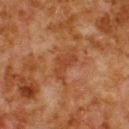Captured during whole-body skin photography for melanoma surveillance; the lesion was not biopsied.
A male patient roughly 80 years of age.
The tile uses cross-polarized illumination.
Approximately 4.5 mm at its widest.
The total-body-photography lesion software estimated internal color variation of about 2.5 on a 0–10 scale and radial color variation of about 1.
The lesion is located on the upper back.
A 15 mm close-up tile from a total-body photography series done for melanoma screening.